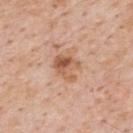Clinical impression: Part of a total-body skin-imaging series; this lesion was reviewed on a skin check and was not flagged for biopsy. Acquisition and patient details: Measured at roughly 3.5 mm in maximum diameter. From the upper back. A male patient, aged approximately 60. Cropped from a total-body skin-imaging series; the visible field is about 15 mm.